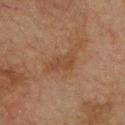Imaged during a routine full-body skin examination; the lesion was not biopsied and no histopathology is available.
Imaged with cross-polarized lighting.
From the chest.
A male patient, aged approximately 75.
This image is a 15 mm lesion crop taken from a total-body photograph.
An algorithmic analysis of the crop reported a lesion area of about 6 mm² and an outline eccentricity of about 0.85 (0 = round, 1 = elongated). The software also gave a mean CIELAB color near L≈37 a*≈18 b*≈28, a lesion–skin lightness drop of about 5, and a lesion-to-skin contrast of about 5.5 (normalized; higher = more distinct). It also reported a within-lesion color-variation index near 2/10 and peripheral color asymmetry of about 1.